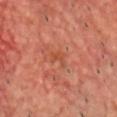No biopsy was performed on this lesion — it was imaged during a full skin examination and was not determined to be concerning.
The lesion is on the chest.
A lesion tile, about 15 mm wide, cut from a 3D total-body photograph.
Measured at roughly 2.5 mm in maximum diameter.
The subject is a male aged approximately 50.
Automated tile analysis of the lesion measured an area of roughly 3 mm² and two-axis asymmetry of about 0.5. The software also gave a mean CIELAB color near L≈50 a*≈30 b*≈35 and about 6 CIELAB-L* units darker than the surrounding skin. The analysis additionally found border irregularity of about 5.5 on a 0–10 scale and a within-lesion color-variation index near 0/10. The analysis additionally found a classifier nevus-likeness of about 0/100 and lesion-presence confidence of about 100/100.